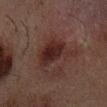  biopsy_status: not biopsied; imaged during a skin examination
  automated_metrics:
    area_mm2_approx: 14.0
    shape_asymmetry: 0.4
    lesion_detection_confidence_0_100: 100
  image:
    source: total-body photography crop
    field_of_view_mm: 15
  lesion_size:
    long_diameter_mm_approx: 5.0
  site: mid back
  patient:
    sex: male
    age_approx: 50
  lighting: cross-polarized